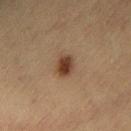lesion size: ≈2.5 mm | location: the left thigh | patient: female, approximately 40 years of age | image: 15 mm crop, total-body photography.